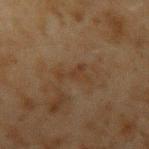Impression:
This lesion was catalogued during total-body skin photography and was not selected for biopsy.
Clinical summary:
A male patient aged 43–47. Located on the left upper arm. Approximately 4 mm at its widest. Automated tile analysis of the lesion measured an average lesion color of about L≈30 a*≈14 b*≈25 (CIELAB), a lesion–skin lightness drop of about 4, and a lesion-to-skin contrast of about 5.5 (normalized; higher = more distinct). A 15 mm crop from a total-body photograph taken for skin-cancer surveillance. Captured under cross-polarized illumination.